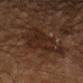Clinical impression: The lesion was photographed on a routine skin check and not biopsied; there is no pathology result. Background: Imaged with cross-polarized lighting. From the left thigh. A male subject, aged approximately 80. Cropped from a total-body skin-imaging series; the visible field is about 15 mm. The lesion-visualizer software estimated an area of roughly 22 mm², a shape eccentricity near 0.7, and two-axis asymmetry of about 0.35.